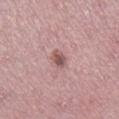Notes:
* follow-up: catalogued during a skin exam; not biopsied
* lesion size: about 3 mm
* tile lighting: white-light
* automated metrics: about 12 CIELAB-L* units darker than the surrounding skin and a normalized lesion–skin contrast near 8; a nevus-likeness score of about 45/100
* subject: female, roughly 45 years of age
* location: the right lower leg
* acquisition: total-body-photography crop, ~15 mm field of view